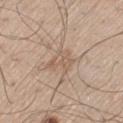No biopsy was performed on this lesion — it was imaged during a full skin examination and was not determined to be concerning. A male patient, aged around 60. A region of skin cropped from a whole-body photographic capture, roughly 15 mm wide. This is a white-light tile. The lesion's longest dimension is about 3.5 mm. Automated image analysis of the tile measured a footprint of about 6 mm², a shape eccentricity near 0.65, and a symmetry-axis asymmetry near 0.4. It also reported a border-irregularity index near 5/10, internal color variation of about 2 on a 0–10 scale, and radial color variation of about 1. The software also gave a nevus-likeness score of about 0/100 and lesion-presence confidence of about 90/100. The lesion is located on the left thigh.